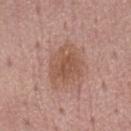<tbp_lesion>
<biopsy_status>not biopsied; imaged during a skin examination</biopsy_status>
<site>abdomen</site>
<image>
  <source>total-body photography crop</source>
  <field_of_view_mm>15</field_of_view_mm>
</image>
<patient>
  <sex>female</sex>
  <age_approx>50</age_approx>
</patient>
</tbp_lesion>